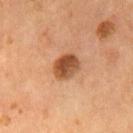This lesion was catalogued during total-body skin photography and was not selected for biopsy.
This is a cross-polarized tile.
A male subject about 55 years old.
The recorded lesion diameter is about 3.5 mm.
From the chest.
A 15 mm crop from a total-body photograph taken for skin-cancer surveillance.